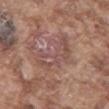<case>
<biopsy_status>not biopsied; imaged during a skin examination</biopsy_status>
<patient>
  <sex>male</sex>
  <age_approx>75</age_approx>
</patient>
<site>abdomen</site>
<image>
  <source>total-body photography crop</source>
  <field_of_view_mm>15</field_of_view_mm>
</image>
</case>